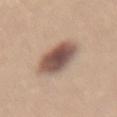<lesion>
  <biopsy_status>not biopsied; imaged during a skin examination</biopsy_status>
  <lighting>white-light</lighting>
  <image>
    <source>total-body photography crop</source>
    <field_of_view_mm>15</field_of_view_mm>
  </image>
  <patient>
    <sex>female</sex>
    <age_approx>25</age_approx>
  </patient>
  <site>lower back</site>
  <lesion_size>
    <long_diameter_mm_approx>5.5</long_diameter_mm_approx>
  </lesion_size>
</lesion>